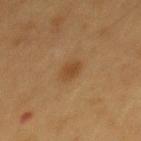biopsy status: catalogued during a skin exam; not biopsied
anatomic site: the mid back
image: 15 mm crop, total-body photography
patient: male, approximately 55 years of age
automated metrics: an area of roughly 4 mm², an eccentricity of roughly 0.65, and two-axis asymmetry of about 0.2; a classifier nevus-likeness of about 85/100 and a lesion-detection confidence of about 100/100